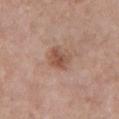workup=total-body-photography surveillance lesion; no biopsy
acquisition=15 mm crop, total-body photography
subject=female, in their mid- to late 50s
body site=the chest
lesion diameter=≈3 mm
tile lighting=white-light illumination